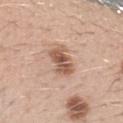Captured during whole-body skin photography for melanoma surveillance; the lesion was not biopsied.
About 4 mm across.
A male subject aged approximately 30.
Cropped from a total-body skin-imaging series; the visible field is about 15 mm.
On the left forearm.